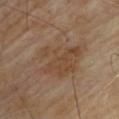Case summary:
* notes — total-body-photography surveillance lesion; no biopsy
* TBP lesion metrics — a nevus-likeness score of about 0/100 and lesion-presence confidence of about 100/100
* patient — male, aged around 65
* site — the upper back
* image — 15 mm crop, total-body photography
* size — about 7 mm
* illumination — cross-polarized